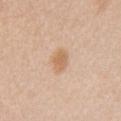Imaged with white-light lighting. Cropped from a whole-body photographic skin survey; the tile spans about 15 mm. On the chest. Longest diameter approximately 3 mm. The patient is a female roughly 45 years of age.